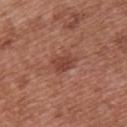Assessment: This lesion was catalogued during total-body skin photography and was not selected for biopsy. Context: Automated image analysis of the tile measured a lesion area of about 4.5 mm², a shape eccentricity near 0.75, and a symmetry-axis asymmetry near 0.25. It also reported a lesion color around L≈43 a*≈25 b*≈28 in CIELAB, a lesion–skin lightness drop of about 9, and a lesion-to-skin contrast of about 7 (normalized; higher = more distinct). The analysis additionally found a nevus-likeness score of about 70/100. The lesion's longest dimension is about 3 mm. A female subject in their 40s. From the upper back. A 15 mm close-up extracted from a 3D total-body photography capture. This is a white-light tile.